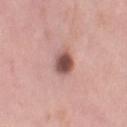The lesion was tiled from a total-body skin photograph and was not biopsied.
A female patient, aged 48 to 52.
This is a white-light tile.
From the leg.
A close-up tile cropped from a whole-body skin photograph, about 15 mm across.
The total-body-photography lesion software estimated roughly 17 lightness units darker than nearby skin and a normalized border contrast of about 11.
Longest diameter approximately 3 mm.